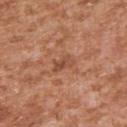Assessment: Captured during whole-body skin photography for melanoma surveillance; the lesion was not biopsied. Image and clinical context: The lesion is on the right upper arm. Approximately 3 mm at its widest. A male subject, in their mid-40s. This image is a 15 mm lesion crop taken from a total-body photograph.